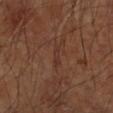Assessment: Recorded during total-body skin imaging; not selected for excision or biopsy. Image and clinical context: A male patient, in their 70s. About 2.5 mm across. Automated image analysis of the tile measured a mean CIELAB color near L≈32 a*≈19 b*≈25, roughly 4 lightness units darker than nearby skin, and a lesion-to-skin contrast of about 4.5 (normalized; higher = more distinct). The software also gave a classifier nevus-likeness of about 0/100 and a lesion-detection confidence of about 65/100. On the left forearm. Captured under cross-polarized illumination. A roughly 15 mm field-of-view crop from a total-body skin photograph.